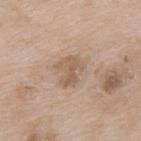Q: Was this lesion biopsied?
A: imaged on a skin check; not biopsied
Q: What is the imaging modality?
A: ~15 mm tile from a whole-body skin photo
Q: Patient demographics?
A: male, approximately 65 years of age
Q: Lesion location?
A: the upper back
Q: How large is the lesion?
A: about 3 mm
Q: Illumination type?
A: white-light